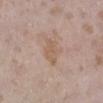| key | value |
|---|---|
| follow-up | catalogued during a skin exam; not biopsied |
| imaging modality | ~15 mm tile from a whole-body skin photo |
| site | the right lower leg |
| automated metrics | a border-irregularity rating of about 2.5/10; a nevus-likeness score of about 0/100 |
| lesion diameter | ~3.5 mm (longest diameter) |
| subject | female, approximately 25 years of age |